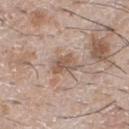On the chest. The subject is a male approximately 65 years of age. Measured at roughly 3 mm in maximum diameter. The tile uses white-light illumination. Automated image analysis of the tile measured a lesion color around L≈55 a*≈16 b*≈26 in CIELAB and a normalized border contrast of about 7. A 15 mm close-up extracted from a 3D total-body photography capture.